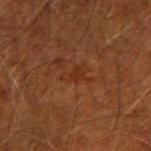Captured during whole-body skin photography for melanoma surveillance; the lesion was not biopsied.